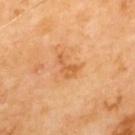image: total-body-photography crop, ~15 mm field of view
diameter: about 3 mm
tile lighting: cross-polarized illumination
anatomic site: the back
image-analysis metrics: a footprint of about 4 mm², an eccentricity of roughly 0.8, and a shape-asymmetry score of about 0.5 (0 = symmetric); a mean CIELAB color near L≈59 a*≈26 b*≈44 and a lesion–skin lightness drop of about 8; a nevus-likeness score of about 15/100 and a detector confidence of about 100 out of 100 that the crop contains a lesion
patient: male, roughly 70 years of age